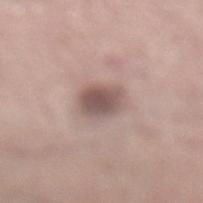<record>
  <image>
    <source>total-body photography crop</source>
    <field_of_view_mm>15</field_of_view_mm>
  </image>
  <patient>
    <sex>male</sex>
    <age_approx>55</age_approx>
  </patient>
  <lesion_size>
    <long_diameter_mm_approx>3.5</long_diameter_mm_approx>
  </lesion_size>
  <lighting>white-light</lighting>
  <site>left lower leg</site>
</record>